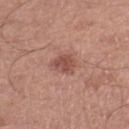Impression:
Recorded during total-body skin imaging; not selected for excision or biopsy.